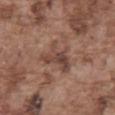| key | value |
|---|---|
| follow-up | total-body-photography surveillance lesion; no biopsy |
| patient | male, approximately 75 years of age |
| image-analysis metrics | a lesion–skin lightness drop of about 9 and a normalized lesion–skin contrast near 7; a classifier nevus-likeness of about 0/100 and a lesion-detection confidence of about 100/100 |
| location | the abdomen |
| illumination | white-light |
| lesion size | ~4.5 mm (longest diameter) |
| imaging modality | 15 mm crop, total-body photography |